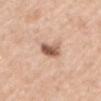Approximately 3 mm at its widest.
This is a white-light tile.
The lesion is located on the front of the torso.
The lesion-visualizer software estimated a lesion area of about 6 mm² and a shape-asymmetry score of about 0.3 (0 = symmetric). The analysis additionally found a mean CIELAB color near L≈58 a*≈20 b*≈29 and a lesion-to-skin contrast of about 9.5 (normalized; higher = more distinct). The analysis additionally found a border-irregularity rating of about 3/10, a color-variation rating of about 6/10, and a peripheral color-asymmetry measure near 2.5.
A male patient, approximately 70 years of age.
A lesion tile, about 15 mm wide, cut from a 3D total-body photograph.